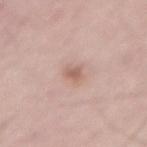{"biopsy_status": "not biopsied; imaged during a skin examination", "site": "lower back", "patient": {"sex": "male", "age_approx": 65}, "image": {"source": "total-body photography crop", "field_of_view_mm": 15}}